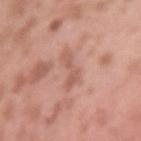  image:
    source: total-body photography crop
    field_of_view_mm: 15
  patient:
    sex: male
    age_approx: 40
  lighting: white-light
  site: left upper arm